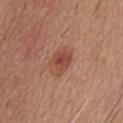Clinical impression: No biopsy was performed on this lesion — it was imaged during a full skin examination and was not determined to be concerning. Context: On the front of the torso. Automated tile analysis of the lesion measured an average lesion color of about L≈47 a*≈25 b*≈29 (CIELAB) and about 10 CIELAB-L* units darker than the surrounding skin. It also reported an automated nevus-likeness rating near 95 out of 100 and lesion-presence confidence of about 100/100. A lesion tile, about 15 mm wide, cut from a 3D total-body photograph. Imaged with white-light lighting. Measured at roughly 3.5 mm in maximum diameter. The patient is a male aged 58 to 62.